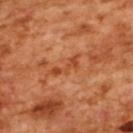biopsy status — no biopsy performed (imaged during a skin exam) | anatomic site — the upper back | image source — ~15 mm tile from a whole-body skin photo | patient — female, approximately 55 years of age.